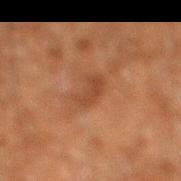biopsy_status: not biopsied; imaged during a skin examination
site: leg
patient:
  sex: male
  age_approx: 60
image:
  source: total-body photography crop
  field_of_view_mm: 15